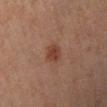- workup: imaged on a skin check; not biopsied